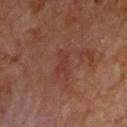Recorded during total-body skin imaging; not selected for excision or biopsy. About 3.5 mm across. From the upper back. The total-body-photography lesion software estimated a lesion area of about 4.5 mm², a shape eccentricity near 0.85, and a symmetry-axis asymmetry near 0.4. It also reported border irregularity of about 5.5 on a 0–10 scale and a color-variation rating of about 1/10. The software also gave a classifier nevus-likeness of about 0/100 and a detector confidence of about 100 out of 100 that the crop contains a lesion. The subject is a male aged approximately 70. A lesion tile, about 15 mm wide, cut from a 3D total-body photograph. The tile uses cross-polarized illumination.